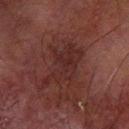Q: Was this lesion biopsied?
A: total-body-photography surveillance lesion; no biopsy
Q: Lesion size?
A: ≈6.5 mm
Q: What kind of image is this?
A: ~15 mm tile from a whole-body skin photo
Q: What are the patient's age and sex?
A: male, approximately 70 years of age
Q: What is the anatomic site?
A: the right forearm
Q: How was the tile lit?
A: cross-polarized illumination